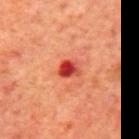Background: A 15 mm crop from a total-body photograph taken for skin-cancer surveillance. On the mid back. Captured under cross-polarized illumination. A male patient aged around 70. Measured at roughly 2.5 mm in maximum diameter.